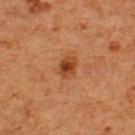Captured during whole-body skin photography for melanoma surveillance; the lesion was not biopsied. A male patient aged 63 to 67. A close-up tile cropped from a whole-body skin photograph, about 15 mm across. The lesion is on the back.